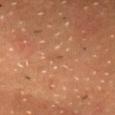lighting: cross-polarized
site: head or neck
image:
  source: total-body photography crop
  field_of_view_mm: 15
patient:
  sex: male
  age_approx: 65